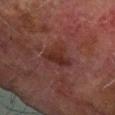The lesion was photographed on a routine skin check and not biopsied; there is no pathology result.
Captured under cross-polarized illumination.
The patient is a male about 75 years old.
On the left forearm.
A 15 mm close-up tile from a total-body photography series done for melanoma screening.